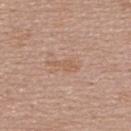notes=no biopsy performed (imaged during a skin exam)
lighting=white-light illumination
anatomic site=the upper back
patient=female, aged around 60
image-analysis metrics=a mean CIELAB color near L≈58 a*≈19 b*≈31 and about 6 CIELAB-L* units darker than the surrounding skin; an automated nevus-likeness rating near 0 out of 100 and a lesion-detection confidence of about 100/100
lesion size=≈3.5 mm
acquisition=total-body-photography crop, ~15 mm field of view